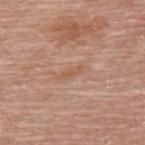Part of a total-body skin-imaging series; this lesion was reviewed on a skin check and was not flagged for biopsy.
The subject is a male aged approximately 80.
About 2.5 mm across.
A region of skin cropped from a whole-body photographic capture, roughly 15 mm wide.
An algorithmic analysis of the crop reported a footprint of about 2 mm², an outline eccentricity of about 0.95 (0 = round, 1 = elongated), and two-axis asymmetry of about 0.4. The software also gave an average lesion color of about L≈56 a*≈21 b*≈32 (CIELAB) and a lesion-to-skin contrast of about 5.5 (normalized; higher = more distinct). The software also gave a nevus-likeness score of about 0/100.
From the upper back.